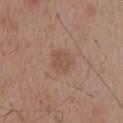Assessment: Captured during whole-body skin photography for melanoma surveillance; the lesion was not biopsied. Context: About 2.5 mm across. A roughly 15 mm field-of-view crop from a total-body skin photograph. The lesion is on the mid back. This is a white-light tile. A male subject roughly 55 years of age.